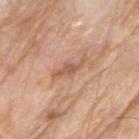Impression:
No biopsy was performed on this lesion — it was imaged during a full skin examination and was not determined to be concerning.
Context:
The subject is a male about 80 years old. On the arm. A 15 mm close-up extracted from a 3D total-body photography capture.